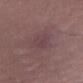Part of a total-body skin-imaging series; this lesion was reviewed on a skin check and was not flagged for biopsy.
A roughly 15 mm field-of-view crop from a total-body skin photograph.
About 4 mm across.
The patient is a male about 70 years old.
The lesion is on the left thigh.
Imaged with white-light lighting.